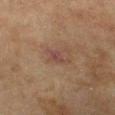  biopsy_status: not biopsied; imaged during a skin examination
  patient:
    sex: female
    age_approx: 80
  lighting: cross-polarized
  site: right forearm
  image:
    source: total-body photography crop
    field_of_view_mm: 15